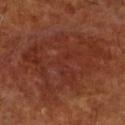workup — no biopsy performed (imaged during a skin exam); site — the right lower leg; patient — male, roughly 60 years of age; acquisition — 15 mm crop, total-body photography.